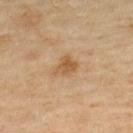Q: What are the patient's age and sex?
A: male, in their mid-40s
Q: How large is the lesion?
A: about 2.5 mm
Q: What is the anatomic site?
A: the upper back
Q: How was the tile lit?
A: cross-polarized illumination
Q: What kind of image is this?
A: ~15 mm crop, total-body skin-cancer survey